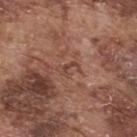Assessment:
Part of a total-body skin-imaging series; this lesion was reviewed on a skin check and was not flagged for biopsy.
Image and clinical context:
The subject is a male aged around 75. A 15 mm crop from a total-body photograph taken for skin-cancer surveillance. The lesion is on the upper back. About 2.5 mm across.